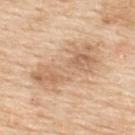Imaged during a routine full-body skin examination; the lesion was not biopsied and no histopathology is available.